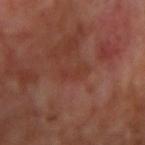Q: Is there a histopathology result?
A: no biopsy performed (imaged during a skin exam)
Q: How large is the lesion?
A: ≈3 mm
Q: What did automated image analysis measure?
A: a footprint of about 3 mm², an outline eccentricity of about 0.95 (0 = round, 1 = elongated), and a shape-asymmetry score of about 0.55 (0 = symmetric); an average lesion color of about L≈35 a*≈24 b*≈27 (CIELAB), about 5 CIELAB-L* units darker than the surrounding skin, and a lesion-to-skin contrast of about 5 (normalized; higher = more distinct); a border-irregularity rating of about 7/10, a within-lesion color-variation index near 0/10, and peripheral color asymmetry of about 0
Q: What lighting was used for the tile?
A: cross-polarized illumination
Q: What are the patient's age and sex?
A: male, aged 68 to 72
Q: What is the anatomic site?
A: the right upper arm
Q: What is the imaging modality?
A: ~15 mm crop, total-body skin-cancer survey